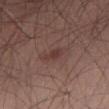{"biopsy_status": "not biopsied; imaged during a skin examination", "site": "abdomen", "automated_metrics": {"color_variation_0_10": 1.0, "peripheral_color_asymmetry": 0.5}, "lighting": "white-light", "patient": {"sex": "male", "age_approx": 50}, "image": {"source": "total-body photography crop", "field_of_view_mm": 15}}